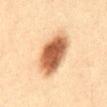Q: Is there a histopathology result?
A: total-body-photography surveillance lesion; no biopsy
Q: What kind of image is this?
A: total-body-photography crop, ~15 mm field of view
Q: What did automated image analysis measure?
A: a border-irregularity rating of about 1.5/10, a color-variation rating of about 6.5/10, and radial color variation of about 1.5
Q: What lighting was used for the tile?
A: cross-polarized illumination
Q: What are the patient's age and sex?
A: male, aged approximately 35
Q: What is the lesion's diameter?
A: ~6 mm (longest diameter)
Q: Where on the body is the lesion?
A: the abdomen